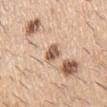Captured during whole-body skin photography for melanoma surveillance; the lesion was not biopsied. The lesion is located on the right upper arm. A male subject aged 58 to 62. A roughly 15 mm field-of-view crop from a total-body skin photograph.